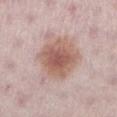{
  "biopsy_status": "not biopsied; imaged during a skin examination",
  "site": "left lower leg",
  "lighting": "white-light",
  "image": {
    "source": "total-body photography crop",
    "field_of_view_mm": 15
  },
  "lesion_size": {
    "long_diameter_mm_approx": 5.0
  },
  "automated_metrics": {
    "area_mm2_approx": 20.0,
    "eccentricity": 0.3,
    "shape_asymmetry": 0.2
  },
  "patient": {
    "sex": "female",
    "age_approx": 30
  }
}